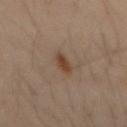<record>
  <biopsy_status>not biopsied; imaged during a skin examination</biopsy_status>
  <image>
    <source>total-body photography crop</source>
    <field_of_view_mm>15</field_of_view_mm>
  </image>
  <site>mid back</site>
  <automated_metrics>
    <cielab_L>42</cielab_L>
    <cielab_a>17</cielab_a>
    <cielab_b>28</cielab_b>
    <vs_skin_contrast_norm>8.5</vs_skin_contrast_norm>
    <color_variation_0_10>2.5</color_variation_0_10>
    <peripheral_color_asymmetry>1.0</peripheral_color_asymmetry>
    <nevus_likeness_0_100>95</nevus_likeness_0_100>
    <lesion_detection_confidence_0_100>100</lesion_detection_confidence_0_100>
  </automated_metrics>
  <lesion_size>
    <long_diameter_mm_approx>3.0</long_diameter_mm_approx>
  </lesion_size>
  <patient>
    <sex>male</sex>
    <age_approx>50</age_approx>
  </patient>
  <lighting>cross-polarized</lighting>
</record>